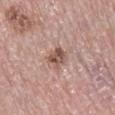The lesion was photographed on a routine skin check and not biopsied; there is no pathology result. A roughly 15 mm field-of-view crop from a total-body skin photograph. Longest diameter approximately 3 mm. On the right lower leg. A male patient aged around 75. The lesion-visualizer software estimated a footprint of about 5 mm² and an outline eccentricity of about 0.7 (0 = round, 1 = elongated). It also reported a lesion color around L≈52 a*≈19 b*≈25 in CIELAB, roughly 12 lightness units darker than nearby skin, and a normalized border contrast of about 8.5. The analysis additionally found a border-irregularity rating of about 2/10, a within-lesion color-variation index near 4/10, and peripheral color asymmetry of about 1.5. It also reported lesion-presence confidence of about 100/100.